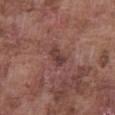Case summary:
- workup · catalogued during a skin exam; not biopsied
- tile lighting · white-light illumination
- patient · male, approximately 75 years of age
- imaging modality · ~15 mm crop, total-body skin-cancer survey
- anatomic site · the front of the torso
- lesion diameter · about 3 mm